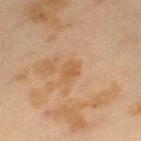{
  "biopsy_status": "not biopsied; imaged during a skin examination",
  "site": "left thigh",
  "image": {
    "source": "total-body photography crop",
    "field_of_view_mm": 15
  },
  "patient": {
    "sex": "female",
    "age_approx": 40
  }
}